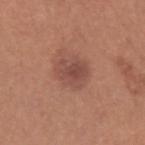Notes:
- follow-up — no biopsy performed (imaged during a skin exam)
- image — ~15 mm tile from a whole-body skin photo
- subject — male, about 40 years old
- location — the left upper arm